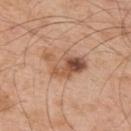biopsy status = total-body-photography surveillance lesion; no biopsy | image = ~15 mm tile from a whole-body skin photo | subject = male, in their mid-50s | location = the upper back.